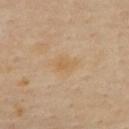Q: Was this lesion biopsied?
A: total-body-photography surveillance lesion; no biopsy
Q: How was this image acquired?
A: total-body-photography crop, ~15 mm field of view
Q: How large is the lesion?
A: about 2.5 mm
Q: What did automated image analysis measure?
A: an area of roughly 3.5 mm², an eccentricity of roughly 0.8, and a symmetry-axis asymmetry near 0.35
Q: What lighting was used for the tile?
A: cross-polarized
Q: Who is the patient?
A: female, aged 58 to 62
Q: Where on the body is the lesion?
A: the upper back